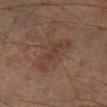Assessment:
The lesion was tiled from a total-body skin photograph and was not biopsied.
Image and clinical context:
The lesion is located on the right lower leg. A region of skin cropped from a whole-body photographic capture, roughly 15 mm wide. A male subject in their 60s. Automated tile analysis of the lesion measured about 6 CIELAB-L* units darker than the surrounding skin and a normalized border contrast of about 5.5. The lesion's longest dimension is about 5.5 mm.